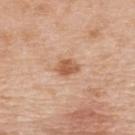Findings:
* biopsy status: imaged on a skin check; not biopsied
* body site: the upper back
* subject: male, in their 70s
* acquisition: total-body-photography crop, ~15 mm field of view
* automated metrics: a border-irregularity index near 2/10
* lesion size: ~3 mm (longest diameter)
* tile lighting: white-light illumination